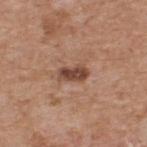The lesion was photographed on a routine skin check and not biopsied; there is no pathology result. Automated image analysis of the tile measured a mean CIELAB color near L≈46 a*≈21 b*≈27, about 13 CIELAB-L* units darker than the surrounding skin, and a normalized lesion–skin contrast near 9.5. And it measured a detector confidence of about 100 out of 100 that the crop contains a lesion. This is a white-light tile. A roughly 15 mm field-of-view crop from a total-body skin photograph. A male patient, roughly 60 years of age. From the upper back.